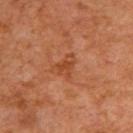Case summary:
- follow-up — total-body-photography surveillance lesion; no biopsy
- image — ~15 mm tile from a whole-body skin photo
- subject — male, aged approximately 60
- tile lighting — cross-polarized
- site — the upper back
- lesion diameter — ≈3 mm